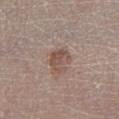{
  "biopsy_status": "not biopsied; imaged during a skin examination",
  "image": {
    "source": "total-body photography crop",
    "field_of_view_mm": 15
  },
  "lesion_size": {
    "long_diameter_mm_approx": 3.0
  },
  "site": "left lower leg",
  "lighting": "white-light",
  "patient": {
    "sex": "female",
    "age_approx": 50
  }
}